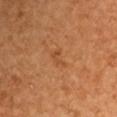Q: Was this lesion biopsied?
A: imaged on a skin check; not biopsied